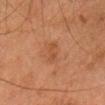Captured during whole-body skin photography for melanoma surveillance; the lesion was not biopsied. A female patient in their 60s. From the head or neck. Cropped from a whole-body photographic skin survey; the tile spans about 15 mm.